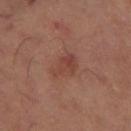A 15 mm close-up tile from a total-body photography series done for melanoma screening. From the left thigh.